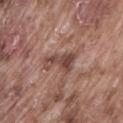Findings:
• workup — imaged on a skin check; not biopsied
• site — the lower back
• automated lesion analysis — a lesion area of about 7 mm² and an outline eccentricity of about 0.8 (0 = round, 1 = elongated); an average lesion color of about L≈45 a*≈20 b*≈24 (CIELAB) and a normalized border contrast of about 8; a border-irregularity index near 6/10, a color-variation rating of about 4/10, and radial color variation of about 1.5; an automated nevus-likeness rating near 0 out of 100
• tile lighting — white-light
• size — ~4 mm (longest diameter)
• image source — ~15 mm tile from a whole-body skin photo
• subject — male, roughly 75 years of age